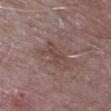Captured during whole-body skin photography for melanoma surveillance; the lesion was not biopsied. From the arm. A male patient, aged approximately 65. Cropped from a total-body skin-imaging series; the visible field is about 15 mm.